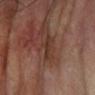Clinical impression:
No biopsy was performed on this lesion — it was imaged during a full skin examination and was not determined to be concerning.
Acquisition and patient details:
About 3 mm across. Imaged with cross-polarized lighting. A male subject, about 70 years old. From the left forearm. A 15 mm close-up extracted from a 3D total-body photography capture.